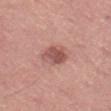Q: Where on the body is the lesion?
A: the left thigh
Q: What is the imaging modality?
A: ~15 mm crop, total-body skin-cancer survey
Q: What is the lesion's diameter?
A: about 3.5 mm
Q: What did automated image analysis measure?
A: a border-irregularity index near 1.5/10, a within-lesion color-variation index near 4.5/10, and radial color variation of about 1.5
Q: Who is the patient?
A: male, aged around 50